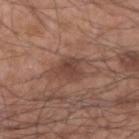Part of a total-body skin-imaging series; this lesion was reviewed on a skin check and was not flagged for biopsy.